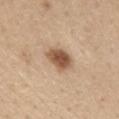biopsy status: no biopsy performed (imaged during a skin exam) | acquisition: 15 mm crop, total-body photography | location: the chest | automated metrics: a lesion area of about 8 mm² and two-axis asymmetry of about 0.25; a lesion-to-skin contrast of about 9.5 (normalized; higher = more distinct); a border-irregularity rating of about 2/10, a color-variation rating of about 4/10, and radial color variation of about 1 | subject: female, aged 58–62.